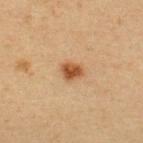biopsy status: total-body-photography surveillance lesion; no biopsy | patient: female, aged 38–42 | tile lighting: cross-polarized illumination | TBP lesion metrics: a lesion area of about 4.5 mm² and a symmetry-axis asymmetry near 0.25 | imaging modality: ~15 mm tile from a whole-body skin photo | size: ~2.5 mm (longest diameter) | anatomic site: the upper back.